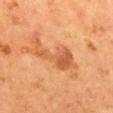follow-up: total-body-photography surveillance lesion; no biopsy
patient: male, approximately 55 years of age
illumination: cross-polarized
image: ~15 mm crop, total-body skin-cancer survey
image-analysis metrics: a lesion area of about 11 mm², an eccentricity of roughly 0.95, and a symmetry-axis asymmetry near 0.65; an automated nevus-likeness rating near 55 out of 100 and lesion-presence confidence of about 100/100
anatomic site: the mid back